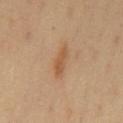<record>
  <biopsy_status>not biopsied; imaged during a skin examination</biopsy_status>
  <lighting>cross-polarized</lighting>
  <lesion_size>
    <long_diameter_mm_approx>4.0</long_diameter_mm_approx>
  </lesion_size>
  <site>chest</site>
  <patient>
    <sex>male</sex>
    <age_approx>40</age_approx>
  </patient>
  <image>
    <source>total-body photography crop</source>
    <field_of_view_mm>15</field_of_view_mm>
  </image>
</record>